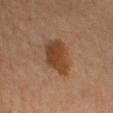Clinical impression:
Imaged during a routine full-body skin examination; the lesion was not biopsied and no histopathology is available.
Context:
The recorded lesion diameter is about 4.5 mm. This image is a 15 mm lesion crop taken from a total-body photograph. This is a cross-polarized tile. A female patient, aged around 40. Automated image analysis of the tile measured two-axis asymmetry of about 0.25. The software also gave an average lesion color of about L≈35 a*≈18 b*≈28 (CIELAB) and about 9 CIELAB-L* units darker than the surrounding skin. The analysis additionally found a border-irregularity index near 2.5/10 and peripheral color asymmetry of about 1. And it measured a nevus-likeness score of about 90/100. The lesion is located on the right lower leg.